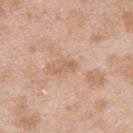Impression: Captured during whole-body skin photography for melanoma surveillance; the lesion was not biopsied. Context: A male subject, in their mid- to late 20s. A close-up tile cropped from a whole-body skin photograph, about 15 mm across. On the upper back.